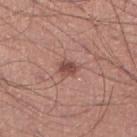follow-up — total-body-photography surveillance lesion; no biopsy | size — ~2.5 mm (longest diameter) | subject — male, approximately 30 years of age | lighting — white-light | acquisition — ~15 mm tile from a whole-body skin photo | anatomic site — the leg.